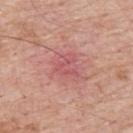{
  "lighting": "white-light",
  "lesion_size": {
    "long_diameter_mm_approx": 2.5
  },
  "image": {
    "source": "total-body photography crop",
    "field_of_view_mm": 15
  },
  "patient": {
    "sex": "male",
    "age_approx": 60
  },
  "automated_metrics": {
    "area_mm2_approx": 4.5,
    "eccentricity": 0.5,
    "shape_asymmetry": 0.6,
    "cielab_L": 55,
    "cielab_a": 31,
    "cielab_b": 23,
    "vs_skin_darker_L": 7.0,
    "border_irregularity_0_10": 8.0,
    "peripheral_color_asymmetry": 0.0
  },
  "site": "upper back"
}